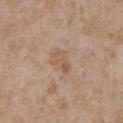No biopsy was performed on this lesion — it was imaged during a full skin examination and was not determined to be concerning.
This is a white-light tile.
A male patient, aged 63–67.
From the chest.
Longest diameter approximately 3.5 mm.
A lesion tile, about 15 mm wide, cut from a 3D total-body photograph.
Automated image analysis of the tile measured an area of roughly 5 mm², an eccentricity of roughly 0.8, and a symmetry-axis asymmetry near 0.35.